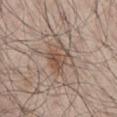follow-up: no biopsy performed (imaged during a skin exam) | diameter: ≈3 mm | image: 15 mm crop, total-body photography | subject: male, in their mid-50s | anatomic site: the abdomen.